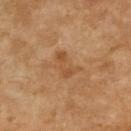Imaged during a routine full-body skin examination; the lesion was not biopsied and no histopathology is available. Measured at roughly 3.5 mm in maximum diameter. On the right upper arm. A 15 mm close-up tile from a total-body photography series done for melanoma screening. A female subject about 70 years old. Captured under cross-polarized illumination.